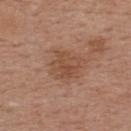Case summary:
– biopsy status: catalogued during a skin exam; not biopsied
– patient: male, roughly 55 years of age
– body site: the upper back
– image: total-body-photography crop, ~15 mm field of view
– lesion diameter: about 3.5 mm
– tile lighting: white-light illumination